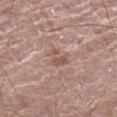  image:
    source: total-body photography crop
    field_of_view_mm: 15
  site: left thigh
  automated_metrics:
    cielab_L: 53
    cielab_a: 19
    cielab_b: 25
    vs_skin_contrast_norm: 6.0
    nevus_likeness_0_100: 0
  patient:
    sex: male
    age_approx: 70